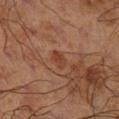The lesion is located on the right lower leg. A 15 mm close-up tile from a total-body photography series done for melanoma screening. A male patient, about 70 years old. The tile uses cross-polarized illumination.